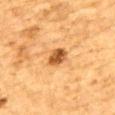Impression:
No biopsy was performed on this lesion — it was imaged during a full skin examination and was not determined to be concerning.
Acquisition and patient details:
A 15 mm crop from a total-body photograph taken for skin-cancer surveillance. A male patient, in their mid- to late 80s or older. The lesion's longest dimension is about 3 mm. The lesion is on the back.